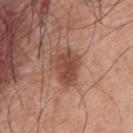Clinical impression: Part of a total-body skin-imaging series; this lesion was reviewed on a skin check and was not flagged for biopsy. Background: Longest diameter approximately 4 mm. The lesion is located on the chest. This is a white-light tile. A male subject aged approximately 70. A close-up tile cropped from a whole-body skin photograph, about 15 mm across.